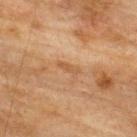Context:
The tile uses cross-polarized illumination. The lesion is on the upper back. The patient is a male approximately 75 years of age. A 15 mm close-up tile from a total-body photography series done for melanoma screening.